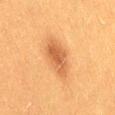The lesion was tiled from a total-body skin photograph and was not biopsied.
The patient is a female aged 38 to 42.
A lesion tile, about 15 mm wide, cut from a 3D total-body photograph.
The lesion is located on the lower back.